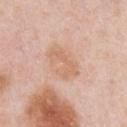Captured during whole-body skin photography for melanoma surveillance; the lesion was not biopsied.
On the chest.
A male subject aged approximately 60.
Captured under white-light illumination.
This image is a 15 mm lesion crop taken from a total-body photograph.
Approximately 4 mm at its widest.